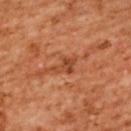Q: Was this lesion biopsied?
A: imaged on a skin check; not biopsied
Q: How large is the lesion?
A: ~3 mm (longest diameter)
Q: Who is the patient?
A: female, aged 53–57
Q: What is the imaging modality?
A: 15 mm crop, total-body photography
Q: Where on the body is the lesion?
A: the upper back